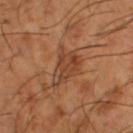Imaged during a routine full-body skin examination; the lesion was not biopsied and no histopathology is available. Automated image analysis of the tile measured a mean CIELAB color near L≈43 a*≈22 b*≈33, roughly 8 lightness units darker than nearby skin, and a lesion-to-skin contrast of about 6.5 (normalized; higher = more distinct). The software also gave a classifier nevus-likeness of about 0/100 and a detector confidence of about 80 out of 100 that the crop contains a lesion. Located on the left thigh. Captured under cross-polarized illumination. A 15 mm crop from a total-body photograph taken for skin-cancer surveillance. Longest diameter approximately 5 mm. The subject is a male aged 63–67.